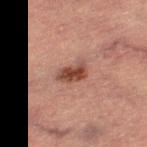Findings:
* workup: total-body-photography surveillance lesion; no biopsy
* subject: female, about 70 years old
* body site: the left thigh
* imaging modality: ~15 mm tile from a whole-body skin photo